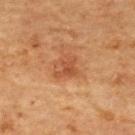{"site": "upper back", "image": {"source": "total-body photography crop", "field_of_view_mm": 15}, "automated_metrics": {"area_mm2_approx": 4.5, "eccentricity": 0.7, "shape_asymmetry": 0.5, "cielab_L": 42, "cielab_a": 24, "cielab_b": 32, "vs_skin_darker_L": 7.0, "vs_skin_contrast_norm": 6.0, "color_variation_0_10": 2.0, "peripheral_color_asymmetry": 0.5}, "patient": {"sex": "female", "age_approx": 70}, "lighting": "cross-polarized"}